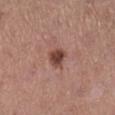Q: Was this lesion biopsied?
A: no biopsy performed (imaged during a skin exam)
Q: Who is the patient?
A: female, roughly 55 years of age
Q: How large is the lesion?
A: about 2.5 mm
Q: Automated lesion metrics?
A: a lesion area of about 5 mm², an eccentricity of roughly 0.35, and two-axis asymmetry of about 0.2
Q: What kind of image is this?
A: total-body-photography crop, ~15 mm field of view
Q: Lesion location?
A: the right lower leg